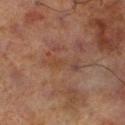Findings:
* notes · catalogued during a skin exam; not biopsied
* acquisition · 15 mm crop, total-body photography
* patient · male, aged 68–72
* lesion size · ~4.5 mm (longest diameter)
* tile lighting · cross-polarized
* automated metrics · an average lesion color of about L≈35 a*≈17 b*≈24 (CIELAB); border irregularity of about 5.5 on a 0–10 scale, a color-variation rating of about 3.5/10, and a peripheral color-asymmetry measure near 0.5; a nevus-likeness score of about 0/100 and lesion-presence confidence of about 100/100
* body site · the left lower leg